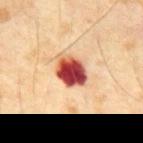Image and clinical context: A male patient, in their mid-60s. A 15 mm crop from a total-body photograph taken for skin-cancer surveillance. Approximately 4 mm at its widest.